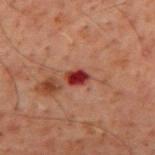Q: Is there a histopathology result?
A: no biopsy performed (imaged during a skin exam)
Q: How was the tile lit?
A: cross-polarized illumination
Q: How was this image acquired?
A: ~15 mm crop, total-body skin-cancer survey
Q: Patient demographics?
A: male, in their 60s
Q: Lesion size?
A: ~2.5 mm (longest diameter)
Q: Where on the body is the lesion?
A: the mid back
Q: Automated lesion metrics?
A: a lesion color around L≈27 a*≈29 b*≈23 in CIELAB, about 14 CIELAB-L* units darker than the surrounding skin, and a normalized border contrast of about 13; a classifier nevus-likeness of about 0/100 and a lesion-detection confidence of about 100/100